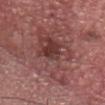Findings:
* biopsy status · imaged on a skin check; not biopsied
* lighting · white-light
* imaging modality · ~15 mm crop, total-body skin-cancer survey
* automated lesion analysis · a lesion color around L≈43 a*≈24 b*≈21 in CIELAB, about 8 CIELAB-L* units darker than the surrounding skin, and a lesion-to-skin contrast of about 6.5 (normalized; higher = more distinct); a border-irregularity rating of about 6/10, a color-variation rating of about 7/10, and peripheral color asymmetry of about 2; lesion-presence confidence of about 95/100
* location · the head or neck
* subject · male, aged 58–62